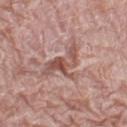The recorded lesion diameter is about 5.5 mm. A female patient, aged around 60. From the left thigh. An algorithmic analysis of the crop reported an average lesion color of about L≈53 a*≈22 b*≈24 (CIELAB), roughly 10 lightness units darker than nearby skin, and a normalized lesion–skin contrast near 7. The analysis additionally found a border-irregularity index near 9/10 and internal color variation of about 8 on a 0–10 scale. It also reported an automated nevus-likeness rating near 15 out of 100 and a detector confidence of about 80 out of 100 that the crop contains a lesion. A region of skin cropped from a whole-body photographic capture, roughly 15 mm wide.